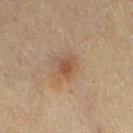{"biopsy_status": "not biopsied; imaged during a skin examination", "lighting": "cross-polarized", "site": "right thigh", "image": {"source": "total-body photography crop", "field_of_view_mm": 15}, "patient": {"sex": "female", "age_approx": 55}}